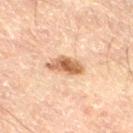biopsy status: no biopsy performed (imaged during a skin exam)
image-analysis metrics: a footprint of about 7 mm², a shape eccentricity near 0.85, and two-axis asymmetry of about 0.3; a lesion color around L≈48 a*≈17 b*≈29 in CIELAB and about 12 CIELAB-L* units darker than the surrounding skin; border irregularity of about 3 on a 0–10 scale and radial color variation of about 1.5; a nevus-likeness score of about 90/100 and lesion-presence confidence of about 100/100
site: the right thigh
patient: male, about 60 years old
acquisition: ~15 mm crop, total-body skin-cancer survey
size: about 4 mm
illumination: cross-polarized illumination